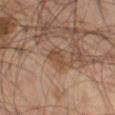Q: Was this lesion biopsied?
A: imaged on a skin check; not biopsied
Q: What is the lesion's diameter?
A: ~2.5 mm (longest diameter)
Q: Where on the body is the lesion?
A: the leg
Q: Illumination type?
A: cross-polarized illumination
Q: What kind of image is this?
A: ~15 mm crop, total-body skin-cancer survey
Q: Who is the patient?
A: male, approximately 55 years of age